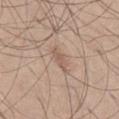Captured during whole-body skin photography for melanoma surveillance; the lesion was not biopsied. Measured at roughly 2.5 mm in maximum diameter. A region of skin cropped from a whole-body photographic capture, roughly 15 mm wide. On the leg. A male subject in their mid-20s. Imaged with white-light lighting.